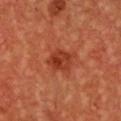This lesion was catalogued during total-body skin photography and was not selected for biopsy. About 3 mm across. A female subject, aged 43 to 47. On the chest. Captured under cross-polarized illumination. A region of skin cropped from a whole-body photographic capture, roughly 15 mm wide. The total-body-photography lesion software estimated a border-irregularity index near 2/10 and a within-lesion color-variation index near 5/10.